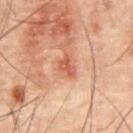Q: Is there a histopathology result?
A: total-body-photography surveillance lesion; no biopsy
Q: Illumination type?
A: cross-polarized
Q: Lesion location?
A: the abdomen
Q: What kind of image is this?
A: ~15 mm crop, total-body skin-cancer survey
Q: Patient demographics?
A: male, approximately 60 years of age
Q: Automated lesion metrics?
A: an area of roughly 4 mm²; border irregularity of about 3.5 on a 0–10 scale, a color-variation rating of about 2.5/10, and a peripheral color-asymmetry measure near 1; an automated nevus-likeness rating near 0 out of 100